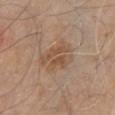follow-up: imaged on a skin check; not biopsied | automated lesion analysis: a lesion area of about 15 mm², a shape eccentricity near 0.6, and a shape-asymmetry score of about 0.2 (0 = symmetric); a border-irregularity rating of about 2.5/10 and internal color variation of about 4.5 on a 0–10 scale; an automated nevus-likeness rating near 0 out of 100 and a detector confidence of about 100 out of 100 that the crop contains a lesion | size: about 4.5 mm | anatomic site: the left upper arm | tile lighting: white-light illumination | image: total-body-photography crop, ~15 mm field of view | subject: male, approximately 80 years of age.